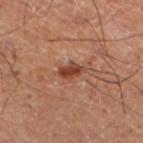Impression: The lesion was photographed on a routine skin check and not biopsied; there is no pathology result. Acquisition and patient details: A 15 mm close-up extracted from a 3D total-body photography capture. The lesion is on the left lower leg. Captured under cross-polarized illumination. A male subject aged 48 to 52. Automated tile analysis of the lesion measured a footprint of about 4.5 mm², an eccentricity of roughly 0.8, and two-axis asymmetry of about 0.3. And it measured a color-variation rating of about 3/10 and a peripheral color-asymmetry measure near 1. The recorded lesion diameter is about 3 mm.